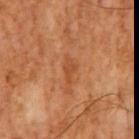{
  "biopsy_status": "not biopsied; imaged during a skin examination",
  "automated_metrics": {
    "vs_skin_darker_L": 7.0,
    "vs_skin_contrast_norm": 5.5
  },
  "lesion_size": {
    "long_diameter_mm_approx": 2.5
  },
  "image": {
    "source": "total-body photography crop",
    "field_of_view_mm": 15
  },
  "patient": {
    "sex": "male",
    "age_approx": 65
  }
}